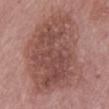The lesion was photographed on a routine skin check and not biopsied; there is no pathology result.
Automated image analysis of the tile measured a footprint of about 75 mm², a shape eccentricity near 0.75, and a shape-asymmetry score of about 0.25 (0 = symmetric). It also reported a classifier nevus-likeness of about 5/100.
The subject is a male aged around 75.
Captured under white-light illumination.
From the abdomen.
A 15 mm close-up extracted from a 3D total-body photography capture.
The recorded lesion diameter is about 11.5 mm.